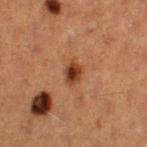Impression: The lesion was tiled from a total-body skin photograph and was not biopsied. Context: A female subject in their 40s. Cropped from a total-body skin-imaging series; the visible field is about 15 mm. Imaged with cross-polarized lighting. Located on the left thigh. About 2.5 mm across.